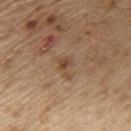follow-up — imaged on a skin check; not biopsied | image source — ~15 mm crop, total-body skin-cancer survey | anatomic site — the upper back | illumination — white-light illumination | subject — male, aged approximately 70.